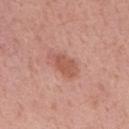Impression: This lesion was catalogued during total-body skin photography and was not selected for biopsy. Image and clinical context: The tile uses white-light illumination. A male subject, roughly 55 years of age. Located on the back. Automated tile analysis of the lesion measured an area of roughly 6.5 mm², a shape eccentricity near 0.85, and a shape-asymmetry score of about 0.25 (0 = symmetric). And it measured roughly 9 lightness units darker than nearby skin and a normalized border contrast of about 6.5. The software also gave a border-irregularity rating of about 3/10, internal color variation of about 2 on a 0–10 scale, and a peripheral color-asymmetry measure near 0.5. The software also gave an automated nevus-likeness rating near 40 out of 100 and a lesion-detection confidence of about 100/100. Measured at roughly 4 mm in maximum diameter. Cropped from a total-body skin-imaging series; the visible field is about 15 mm.